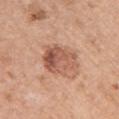Q: Was this lesion biopsied?
A: catalogued during a skin exam; not biopsied